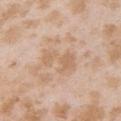Findings:
• biopsy status · no biopsy performed (imaged during a skin exam)
• diameter · ≈4 mm
• TBP lesion metrics · an area of roughly 8 mm²; a detector confidence of about 100 out of 100 that the crop contains a lesion
• imaging modality · 15 mm crop, total-body photography
• patient · female, approximately 25 years of age
• lighting · white-light illumination
• location · the left upper arm